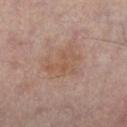Recorded during total-body skin imaging; not selected for excision or biopsy. A 15 mm crop from a total-body photograph taken for skin-cancer surveillance. The lesion is on the left leg. About 4.5 mm across. This is a cross-polarized tile. The subject is a male in their 50s.